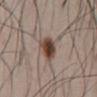Assessment: Part of a total-body skin-imaging series; this lesion was reviewed on a skin check and was not flagged for biopsy. Context: A male patient, about 65 years old. Captured under white-light illumination. A 15 mm close-up extracted from a 3D total-body photography capture. Measured at roughly 3 mm in maximum diameter. On the chest. An algorithmic analysis of the crop reported an average lesion color of about L≈43 a*≈16 b*≈23 (CIELAB), roughly 15 lightness units darker than nearby skin, and a normalized lesion–skin contrast near 11.5. It also reported radial color variation of about 2.5.